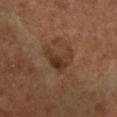workup: total-body-photography surveillance lesion; no biopsy
patient: female, about 65 years old
acquisition: ~15 mm tile from a whole-body skin photo
tile lighting: cross-polarized
site: the leg